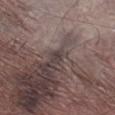Diagnosis:
The lesion was biopsied, and histopathology showed an invasive melanoma; Breslow depth 0.2 mm — a malignant lesion.